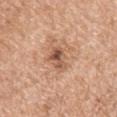This lesion was catalogued during total-body skin photography and was not selected for biopsy.
A 15 mm crop from a total-body photograph taken for skin-cancer surveillance.
The patient is a female aged approximately 70.
From the left upper arm.
The lesion's longest dimension is about 3 mm.
Captured under white-light illumination.